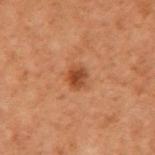Impression: Captured during whole-body skin photography for melanoma surveillance; the lesion was not biopsied. Context: Located on the arm. The lesion's longest dimension is about 2.5 mm. A male patient, in their 70s. Cropped from a whole-body photographic skin survey; the tile spans about 15 mm.